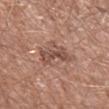{
  "biopsy_status": "not biopsied; imaged during a skin examination",
  "lesion_size": {
    "long_diameter_mm_approx": 4.0
  },
  "patient": {
    "sex": "male",
    "age_approx": 60
  },
  "image": {
    "source": "total-body photography crop",
    "field_of_view_mm": 15
  },
  "lighting": "white-light",
  "site": "left lower leg",
  "automated_metrics": {
    "border_irregularity_0_10": 6.5,
    "color_variation_0_10": 3.5
  }
}